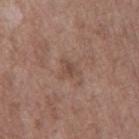| feature | finding |
|---|---|
| notes | no biopsy performed (imaged during a skin exam) |
| subject | male, about 70 years old |
| imaging modality | 15 mm crop, total-body photography |
| location | the back |
| illumination | white-light illumination |